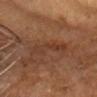Impression: No biopsy was performed on this lesion — it was imaged during a full skin examination and was not determined to be concerning. Clinical summary: Located on the head or neck. The tile uses cross-polarized illumination. A 15 mm crop from a total-body photograph taken for skin-cancer surveillance. The subject is a male approximately 60 years of age.